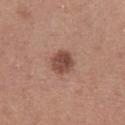biopsy_status: not biopsied; imaged during a skin examination
lighting: white-light
lesion_size:
  long_diameter_mm_approx: 3.0
site: chest
patient:
  sex: female
  age_approx: 50
image:
  source: total-body photography crop
  field_of_view_mm: 15